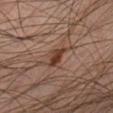<lesion>
  <biopsy_status>not biopsied; imaged during a skin examination</biopsy_status>
  <lighting>cross-polarized</lighting>
  <patient>
    <sex>male</sex>
    <age_approx>45</age_approx>
  </patient>
  <image>
    <source>total-body photography crop</source>
    <field_of_view_mm>15</field_of_view_mm>
  </image>
  <automated_metrics>
    <area_mm2_approx>4.0</area_mm2_approx>
    <eccentricity>0.8</eccentricity>
    <shape_asymmetry>0.4</shape_asymmetry>
    <border_irregularity_0_10>3.5</border_irregularity_0_10>
    <nevus_likeness_0_100>90</nevus_likeness_0_100>
    <lesion_detection_confidence_0_100>100</lesion_detection_confidence_0_100>
  </automated_metrics>
  <site>left lower leg</site>
  <lesion_size>
    <long_diameter_mm_approx>3.0</long_diameter_mm_approx>
  </lesion_size>
</lesion>